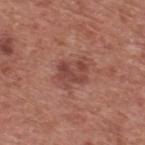<tbp_lesion>
<biopsy_status>not biopsied; imaged during a skin examination</biopsy_status>
<lighting>white-light</lighting>
<lesion_size>
  <long_diameter_mm_approx>3.5</long_diameter_mm_approx>
</lesion_size>
<site>upper back</site>
<image>
  <source>total-body photography crop</source>
  <field_of_view_mm>15</field_of_view_mm>
</image>
<patient>
  <sex>male</sex>
  <age_approx>75</age_approx>
</patient>
</tbp_lesion>